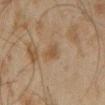Captured during whole-body skin photography for melanoma surveillance; the lesion was not biopsied.